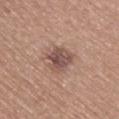Impression:
The lesion was photographed on a routine skin check and not biopsied; there is no pathology result.
Image and clinical context:
A 15 mm close-up tile from a total-body photography series done for melanoma screening. From the upper back. A female patient aged 63–67.